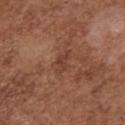– notes — imaged on a skin check; not biopsied
– lighting — white-light
– patient — female, in their mid-70s
– body site — the chest
– acquisition — total-body-photography crop, ~15 mm field of view